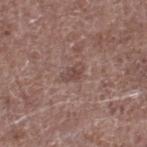{"biopsy_status": "not biopsied; imaged during a skin examination", "patient": {"sex": "male", "age_approx": 70}, "image": {"source": "total-body photography crop", "field_of_view_mm": 15}, "site": "right lower leg", "lesion_size": {"long_diameter_mm_approx": 2.5}}